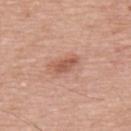This image is a 15 mm lesion crop taken from a total-body photograph. The patient is a male about 55 years old. Imaged with white-light lighting. The recorded lesion diameter is about 3 mm.